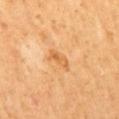The lesion was tiled from a total-body skin photograph and was not biopsied. A male subject, aged around 60. Located on the mid back. A 15 mm close-up extracted from a 3D total-body photography capture.